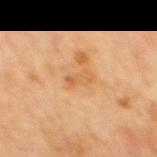Assessment:
The lesion was photographed on a routine skin check and not biopsied; there is no pathology result.
Acquisition and patient details:
Located on the mid back. The patient is a male aged 63 to 67. The lesion's longest dimension is about 3 mm. Captured under cross-polarized illumination. A 15 mm close-up extracted from a 3D total-body photography capture.